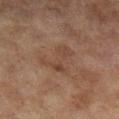Impression:
Part of a total-body skin-imaging series; this lesion was reviewed on a skin check and was not flagged for biopsy.
Context:
The lesion is on the right lower leg. A female subject, roughly 60 years of age. The lesion's longest dimension is about 4 mm. A 15 mm crop from a total-body photograph taken for skin-cancer surveillance.